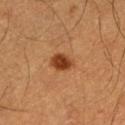On the leg.
A male subject, approximately 60 years of age.
A 15 mm close-up extracted from a 3D total-body photography capture.
This is a cross-polarized tile.
Automated image analysis of the tile measured an area of roughly 5.5 mm², an outline eccentricity of about 0.6 (0 = round, 1 = elongated), and two-axis asymmetry of about 0.1. The software also gave a border-irregularity rating of about 1/10 and peripheral color asymmetry of about 1.
Approximately 3 mm at its widest.